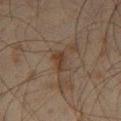No biopsy was performed on this lesion — it was imaged during a full skin examination and was not determined to be concerning. This is a cross-polarized tile. Longest diameter approximately 2.5 mm. The lesion is located on the left thigh. A 15 mm close-up extracted from a 3D total-body photography capture. A male subject, aged 43 to 47.